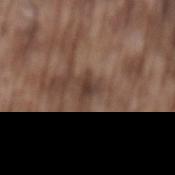biopsy status: no biopsy performed (imaged during a skin exam) | image source: 15 mm crop, total-body photography | automated metrics: a lesion-to-skin contrast of about 8 (normalized; higher = more distinct) | subject: male, aged 73–77 | tile lighting: white-light illumination | lesion diameter: ≈2.5 mm | location: the back.